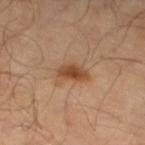Q: Was this lesion biopsied?
A: imaged on a skin check; not biopsied
Q: What is the anatomic site?
A: the left lower leg
Q: What kind of image is this?
A: 15 mm crop, total-body photography
Q: Patient demographics?
A: male, approximately 65 years of age
Q: Lesion size?
A: about 3.5 mm
Q: What lighting was used for the tile?
A: cross-polarized
Q: Automated lesion metrics?
A: a footprint of about 6.5 mm², a shape eccentricity near 0.85, and two-axis asymmetry of about 0.3; a border-irregularity rating of about 3.5/10, a color-variation rating of about 3/10, and radial color variation of about 0.5